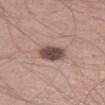The lesion was tiled from a total-body skin photograph and was not biopsied. A 15 mm crop from a total-body photograph taken for skin-cancer surveillance. Imaged with white-light lighting. Located on the left thigh. Approximately 4.5 mm at its widest. A male patient aged around 50. An algorithmic analysis of the crop reported a border-irregularity index near 2/10, a color-variation rating of about 7/10, and radial color variation of about 2. It also reported a classifier nevus-likeness of about 80/100 and lesion-presence confidence of about 100/100.